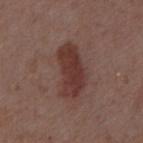This lesion was catalogued during total-body skin photography and was not selected for biopsy. The subject is a male approximately 55 years of age. The tile uses white-light illumination. Automated tile analysis of the lesion measured an eccentricity of roughly 0.9 and a symmetry-axis asymmetry near 0.2. The software also gave a lesion color around L≈35 a*≈21 b*≈22 in CIELAB, roughly 10 lightness units darker than nearby skin, and a lesion-to-skin contrast of about 8.5 (normalized; higher = more distinct). And it measured a border-irregularity index near 3/10, internal color variation of about 3 on a 0–10 scale, and radial color variation of about 1. A 15 mm close-up tile from a total-body photography series done for melanoma screening. Approximately 6 mm at its widest. Located on the abdomen.